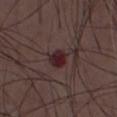follow-up: catalogued during a skin exam; not biopsied
diameter: ~2.5 mm (longest diameter)
TBP lesion metrics: a footprint of about 4.5 mm², an outline eccentricity of about 0.45 (0 = round, 1 = elongated), and a shape-asymmetry score of about 0.15 (0 = symmetric); a lesion color around L≈24 a*≈21 b*≈13 in CIELAB and a lesion–skin lightness drop of about 10
subject: male, roughly 50 years of age
imaging modality: total-body-photography crop, ~15 mm field of view
illumination: white-light illumination